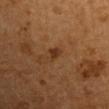follow-up — imaged on a skin check; not biopsied
tile lighting — cross-polarized
patient — male, aged 53 to 57
automated metrics — a lesion color around L≈31 a*≈19 b*≈30 in CIELAB and a lesion–skin lightness drop of about 7; a border-irregularity index near 3/10, a within-lesion color-variation index near 0.5/10, and peripheral color asymmetry of about 0; an automated nevus-likeness rating near 65 out of 100 and a detector confidence of about 100 out of 100 that the crop contains a lesion
location — the arm
image source — ~15 mm tile from a whole-body skin photo
lesion size — about 2.5 mm